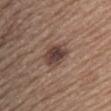– follow-up — catalogued during a skin exam; not biopsied
– tile lighting — white-light illumination
– automated metrics — an outline eccentricity of about 0.5 (0 = round, 1 = elongated); a mean CIELAB color near L≈42 a*≈16 b*≈22, roughly 12 lightness units darker than nearby skin, and a normalized border contrast of about 9.5; a border-irregularity rating of about 2/10 and a peripheral color-asymmetry measure near 1.5; a nevus-likeness score of about 85/100 and a detector confidence of about 100 out of 100 that the crop contains a lesion
– lesion size — about 3.5 mm
– imaging modality — ~15 mm crop, total-body skin-cancer survey
– subject — female, in their mid- to late 60s
– site — the back